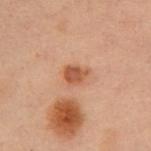The lesion was photographed on a routine skin check and not biopsied; there is no pathology result. A roughly 15 mm field-of-view crop from a total-body skin photograph. Imaged with cross-polarized lighting. The recorded lesion diameter is about 2.5 mm. A female subject, in their mid- to late 50s. Located on the front of the torso.